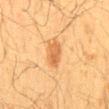{"biopsy_status": "not biopsied; imaged during a skin examination"}